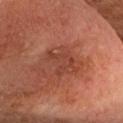Findings:
– biopsy status · imaged on a skin check; not biopsied
– image · ~15 mm tile from a whole-body skin photo
– site · the head or neck
– patient · male, aged 58–62